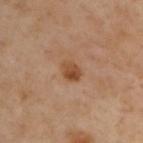The lesion was photographed on a routine skin check and not biopsied; there is no pathology result. Measured at roughly 2.5 mm in maximum diameter. The lesion is located on the back. The tile uses cross-polarized illumination. A male subject, aged 53–57. This image is a 15 mm lesion crop taken from a total-body photograph.